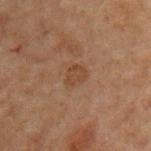Q: Was a biopsy performed?
A: imaged on a skin check; not biopsied
Q: Illumination type?
A: cross-polarized illumination
Q: What kind of image is this?
A: 15 mm crop, total-body photography
Q: How large is the lesion?
A: ≈2.5 mm
Q: Automated lesion metrics?
A: a mean CIELAB color near L≈33 a*≈16 b*≈25, about 5 CIELAB-L* units darker than the surrounding skin, and a normalized lesion–skin contrast near 5.5; a border-irregularity rating of about 2/10 and peripheral color asymmetry of about 0.5
Q: Where on the body is the lesion?
A: the front of the torso
Q: Patient demographics?
A: male, approximately 70 years of age